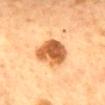<record>
  <biopsy_status>not biopsied; imaged during a skin examination</biopsy_status>
  <image>
    <source>total-body photography crop</source>
    <field_of_view_mm>15</field_of_view_mm>
  </image>
  <site>mid back</site>
  <lesion_size>
    <long_diameter_mm_approx>4.5</long_diameter_mm_approx>
  </lesion_size>
  <patient>
    <sex>female</sex>
    <age_approx>60</age_approx>
  </patient>
  <lighting>cross-polarized</lighting>
</record>